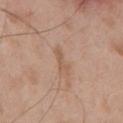TBP lesion metrics: a footprint of about 3.5 mm², an outline eccentricity of about 0.9 (0 = round, 1 = elongated), and two-axis asymmetry of about 0.35; body site: the front of the torso; acquisition: ~15 mm crop, total-body skin-cancer survey; size: about 3.5 mm; patient: male, approximately 65 years of age.